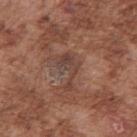<case>
<biopsy_status>not biopsied; imaged during a skin examination</biopsy_status>
<patient>
  <sex>male</sex>
  <age_approx>75</age_approx>
</patient>
<site>right upper arm</site>
<lighting>white-light</lighting>
<lesion_size>
  <long_diameter_mm_approx>4.5</long_diameter_mm_approx>
</lesion_size>
<image>
  <source>total-body photography crop</source>
  <field_of_view_mm>15</field_of_view_mm>
</image>
</case>